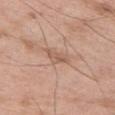Assessment:
No biopsy was performed on this lesion — it was imaged during a full skin examination and was not determined to be concerning.
Clinical summary:
About 3 mm across. An algorithmic analysis of the crop reported an area of roughly 3.5 mm², an outline eccentricity of about 0.8 (0 = round, 1 = elongated), and a shape-asymmetry score of about 0.4 (0 = symmetric). The analysis additionally found a mean CIELAB color near L≈57 a*≈19 b*≈29, about 8 CIELAB-L* units darker than the surrounding skin, and a normalized lesion–skin contrast near 5.5. It also reported an automated nevus-likeness rating near 0 out of 100 and a detector confidence of about 100 out of 100 that the crop contains a lesion. The tile uses white-light illumination. The subject is a male aged approximately 50. A 15 mm close-up extracted from a 3D total-body photography capture. From the left thigh.